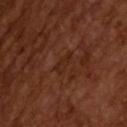follow-up — catalogued during a skin exam; not biopsied | subject — male, roughly 65 years of age | imaging modality — ~15 mm tile from a whole-body skin photo | automated metrics — an area of roughly 3.5 mm², an outline eccentricity of about 0.85 (0 = round, 1 = elongated), and a symmetry-axis asymmetry near 0.35; a border-irregularity index near 3.5/10, a within-lesion color-variation index near 1/10, and a peripheral color-asymmetry measure near 0.5 | illumination — cross-polarized illumination.